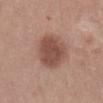{
  "biopsy_status": "not biopsied; imaged during a skin examination",
  "lighting": "white-light",
  "site": "abdomen",
  "image": {
    "source": "total-body photography crop",
    "field_of_view_mm": 15
  },
  "patient": {
    "sex": "female",
    "age_approx": 35
  },
  "lesion_size": {
    "long_diameter_mm_approx": 5.0
  }
}